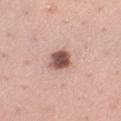Impression:
The lesion was tiled from a total-body skin photograph and was not biopsied.
Context:
A female subject aged 33–37. A lesion tile, about 15 mm wide, cut from a 3D total-body photograph. The lesion is located on the left thigh.